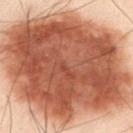This lesion was catalogued during total-body skin photography and was not selected for biopsy.